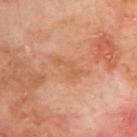This lesion was catalogued during total-body skin photography and was not selected for biopsy. A lesion tile, about 15 mm wide, cut from a 3D total-body photograph. Located on the upper back. The patient is a male approximately 70 years of age.